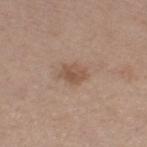Impression: Captured during whole-body skin photography for melanoma surveillance; the lesion was not biopsied. Acquisition and patient details: Located on the right thigh. An algorithmic analysis of the crop reported a footprint of about 5 mm² and an outline eccentricity of about 0.7 (0 = round, 1 = elongated). The software also gave a mean CIELAB color near L≈52 a*≈18 b*≈28 and a lesion-to-skin contrast of about 6.5 (normalized; higher = more distinct). It also reported border irregularity of about 2 on a 0–10 scale, internal color variation of about 3.5 on a 0–10 scale, and radial color variation of about 1. The analysis additionally found a nevus-likeness score of about 35/100 and a detector confidence of about 100 out of 100 that the crop contains a lesion. A 15 mm crop from a total-body photograph taken for skin-cancer surveillance. A female subject, aged 53–57.